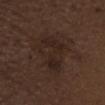A male patient in their 70s.
The lesion's longest dimension is about 5.5 mm.
The lesion is located on the head or neck.
This is a white-light tile.
A lesion tile, about 15 mm wide, cut from a 3D total-body photograph.